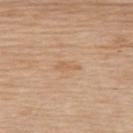Notes:
– workup · total-body-photography surveillance lesion; no biopsy
– patient · female, roughly 65 years of age
– body site · the upper back
– tile lighting · white-light
– diameter · ~3 mm (longest diameter)
– image · total-body-photography crop, ~15 mm field of view